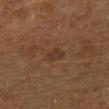A male patient in their mid- to late 60s. A lesion tile, about 15 mm wide, cut from a 3D total-body photograph. Located on the leg.